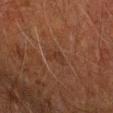Case summary:
– workup — no biopsy performed (imaged during a skin exam)
– image — ~15 mm crop, total-body skin-cancer survey
– diameter — ~2.5 mm (longest diameter)
– site — the left arm
– tile lighting — cross-polarized
– subject — male, roughly 60 years of age
– TBP lesion metrics — a lesion area of about 3.5 mm²; a classifier nevus-likeness of about 0/100 and a lesion-detection confidence of about 90/100